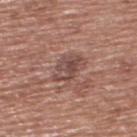- follow-up: catalogued during a skin exam; not biopsied
- image-analysis metrics: border irregularity of about 4 on a 0–10 scale and internal color variation of about 5 on a 0–10 scale; a detector confidence of about 100 out of 100 that the crop contains a lesion
- imaging modality: ~15 mm crop, total-body skin-cancer survey
- tile lighting: white-light illumination
- anatomic site: the back
- patient: male, in their mid-70s